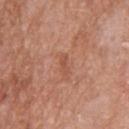{
  "biopsy_status": "not biopsied; imaged during a skin examination",
  "patient": {
    "sex": "female",
    "age_approx": 75
  },
  "site": "back",
  "lighting": "white-light",
  "automated_metrics": {
    "area_mm2_approx": 2.5,
    "eccentricity": 0.9,
    "shape_asymmetry": 0.4,
    "nevus_likeness_0_100": 0,
    "lesion_detection_confidence_0_100": 100
  },
  "lesion_size": {
    "long_diameter_mm_approx": 2.5
  },
  "image": {
    "source": "total-body photography crop",
    "field_of_view_mm": 15
  }
}